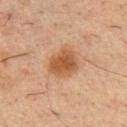The lesion-visualizer software estimated an outline eccentricity of about 0.55 (0 = round, 1 = elongated) and a shape-asymmetry score of about 0.2 (0 = symmetric). It also reported an average lesion color of about L≈50 a*≈22 b*≈34 (CIELAB), roughly 11 lightness units darker than nearby skin, and a normalized lesion–skin contrast near 8.5. It also reported a border-irregularity rating of about 2/10, a within-lesion color-variation index near 3/10, and a peripheral color-asymmetry measure near 1. The software also gave a classifier nevus-likeness of about 95/100 and a detector confidence of about 100 out of 100 that the crop contains a lesion.
The lesion's longest dimension is about 4 mm.
This is a cross-polarized tile.
From the chest.
A close-up tile cropped from a whole-body skin photograph, about 15 mm across.
A male subject about 35 years old.